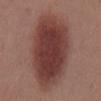Part of a total-body skin-imaging series; this lesion was reviewed on a skin check and was not flagged for biopsy. A 15 mm crop from a total-body photograph taken for skin-cancer surveillance. A male patient, aged around 55. From the mid back. The lesion's longest dimension is about 11 mm. This is a white-light tile.